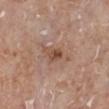The lesion was tiled from a total-body skin photograph and was not biopsied.
Cropped from a whole-body photographic skin survey; the tile spans about 15 mm.
From the left lower leg.
A female subject, about 75 years old.